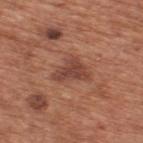Clinical impression:
No biopsy was performed on this lesion — it was imaged during a full skin examination and was not determined to be concerning.
Acquisition and patient details:
Cropped from a whole-body photographic skin survey; the tile spans about 15 mm. The lesion is located on the upper back. The recorded lesion diameter is about 4 mm. A male patient aged around 65.